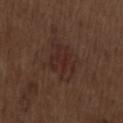workup: imaged on a skin check; not biopsied | image-analysis metrics: an area of roughly 11 mm², an outline eccentricity of about 0.75 (0 = round, 1 = elongated), and a shape-asymmetry score of about 0.25 (0 = symmetric); a lesion color around L≈27 a*≈18 b*≈21 in CIELAB, roughly 5 lightness units darker than nearby skin, and a normalized border contrast of about 6; internal color variation of about 3.5 on a 0–10 scale and a peripheral color-asymmetry measure near 1 | illumination: white-light illumination | patient: male, about 70 years old | image source: ~15 mm crop, total-body skin-cancer survey | site: the lower back | lesion size: about 5 mm.